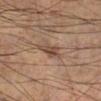biopsy status: catalogued during a skin exam; not biopsied | site: the leg | image source: 15 mm crop, total-body photography | patient: male, about 60 years old | automated metrics: two-axis asymmetry of about 0.25; a mean CIELAB color near L≈45 a*≈17 b*≈26 and a lesion-to-skin contrast of about 8 (normalized; higher = more distinct); an automated nevus-likeness rating near 45 out of 100 and a detector confidence of about 80 out of 100 that the crop contains a lesion.